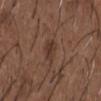This lesion was catalogued during total-body skin photography and was not selected for biopsy.
Automated tile analysis of the lesion measured an automated nevus-likeness rating near 60 out of 100.
The patient is a male aged 48 to 52.
On the chest.
This is a white-light tile.
A 15 mm crop from a total-body photograph taken for skin-cancer surveillance.